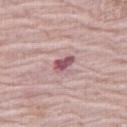Automated tile analysis of the lesion measured a footprint of about 3.5 mm² and an outline eccentricity of about 0.85 (0 = round, 1 = elongated). The analysis additionally found an average lesion color of about L≈53 a*≈27 b*≈15 (CIELAB), about 15 CIELAB-L* units darker than the surrounding skin, and a normalized lesion–skin contrast near 10.5. And it measured a nevus-likeness score of about 5/100. The lesion's longest dimension is about 2.5 mm. The tile uses white-light illumination. On the right thigh. A roughly 15 mm field-of-view crop from a total-body skin photograph. The subject is a female aged 63–67.